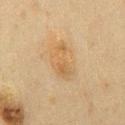This is a cross-polarized tile.
About 5 mm across.
Automated image analysis of the tile measured internal color variation of about 3 on a 0–10 scale and radial color variation of about 1. The software also gave a detector confidence of about 100 out of 100 that the crop contains a lesion.
A close-up tile cropped from a whole-body skin photograph, about 15 mm across.
The lesion is on the chest.
A male subject, aged approximately 60.